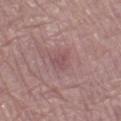biopsy_status: not biopsied; imaged during a skin examination
image:
  source: total-body photography crop
  field_of_view_mm: 15
lighting: white-light
site: left lower leg
lesion_size:
  long_diameter_mm_approx: 3.0
patient:
  sex: female
  age_approx: 70